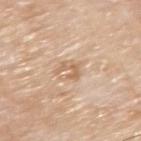The lesion was tiled from a total-body skin photograph and was not biopsied. The lesion is on the arm. Longest diameter approximately 3.5 mm. A region of skin cropped from a whole-body photographic capture, roughly 15 mm wide. The tile uses white-light illumination. A male subject roughly 80 years of age.